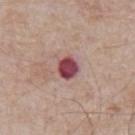Clinical impression:
Recorded during total-body skin imaging; not selected for excision or biopsy.
Image and clinical context:
A male subject, in their mid- to late 60s. The recorded lesion diameter is about 3 mm. Imaged with white-light lighting. The lesion-visualizer software estimated a footprint of about 6 mm², an eccentricity of roughly 0.4, and two-axis asymmetry of about 0.15. The analysis additionally found an average lesion color of about L≈46 a*≈29 b*≈18 (CIELAB) and roughly 17 lightness units darker than nearby skin. It also reported peripheral color asymmetry of about 1. The analysis additionally found an automated nevus-likeness rating near 0 out of 100 and a lesion-detection confidence of about 100/100. The lesion is on the chest. Cropped from a whole-body photographic skin survey; the tile spans about 15 mm.